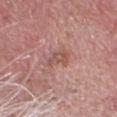| key | value |
|---|---|
| image source | ~15 mm crop, total-body skin-cancer survey |
| site | the head or neck |
| image-analysis metrics | an area of roughly 4 mm², an outline eccentricity of about 0.8 (0 = round, 1 = elongated), and a shape-asymmetry score of about 0.4 (0 = symmetric); a mean CIELAB color near L≈53 a*≈23 b*≈26, roughly 8 lightness units darker than nearby skin, and a lesion-to-skin contrast of about 6 (normalized; higher = more distinct) |
| tile lighting | white-light |
| patient | male, roughly 80 years of age |
| lesion size | about 2.5 mm |